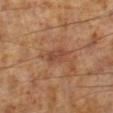The lesion was tiled from a total-body skin photograph and was not biopsied. Approximately 4 mm at its widest. A male patient, about 60 years old. This is a cross-polarized tile. Located on the left lower leg. Cropped from a total-body skin-imaging series; the visible field is about 15 mm. Automated image analysis of the tile measured a footprint of about 6 mm², a shape eccentricity near 0.85, and a symmetry-axis asymmetry near 0.3. And it measured a border-irregularity rating of about 3.5/10 and a color-variation rating of about 2.5/10. The software also gave an automated nevus-likeness rating near 0 out of 100.